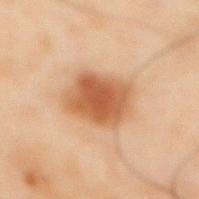Part of a total-body skin-imaging series; this lesion was reviewed on a skin check and was not flagged for biopsy. The subject is a male approximately 50 years of age. The lesion's longest dimension is about 5 mm. A 15 mm crop from a total-body photograph taken for skin-cancer surveillance. The lesion is located on the abdomen. Imaged with cross-polarized lighting.